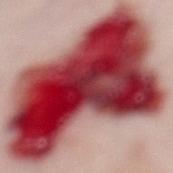Q: What lighting was used for the tile?
A: white-light
Q: How large is the lesion?
A: ≈12 mm
Q: Who is the patient?
A: female, about 50 years old
Q: Lesion location?
A: the left lower leg
Q: How was this image acquired?
A: ~15 mm crop, total-body skin-cancer survey
Q: What did pathology find?
A: a dysplastic (Clark) nevus